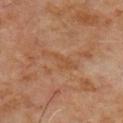* notes — total-body-photography surveillance lesion; no biopsy
* subject — male, aged 58 to 62
* image-analysis metrics — a footprint of about 5.5 mm², an outline eccentricity of about 0.9 (0 = round, 1 = elongated), and a shape-asymmetry score of about 0.5 (0 = symmetric); a border-irregularity index near 6/10, a within-lesion color-variation index near 1/10, and radial color variation of about 0.5
* lesion diameter — ~4.5 mm (longest diameter)
* location — the chest
* illumination — cross-polarized illumination
* image — ~15 mm tile from a whole-body skin photo